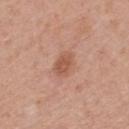<record>
  <biopsy_status>not biopsied; imaged during a skin examination</biopsy_status>
  <site>mid back</site>
  <lesion_size>
    <long_diameter_mm_approx>3.0</long_diameter_mm_approx>
  </lesion_size>
  <patient>
    <sex>male</sex>
    <age_approx>65</age_approx>
  </patient>
  <image>
    <source>total-body photography crop</source>
    <field_of_view_mm>15</field_of_view_mm>
  </image>
</record>